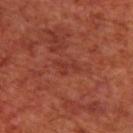This lesion was catalogued during total-body skin photography and was not selected for biopsy.
A male patient, aged approximately 70.
A 15 mm close-up extracted from a 3D total-body photography capture.
Measured at roughly 3 mm in maximum diameter.
On the upper back.